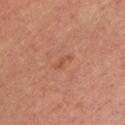No biopsy was performed on this lesion — it was imaged during a full skin examination and was not determined to be concerning. The lesion-visualizer software estimated a lesion area of about 3.5 mm², a shape eccentricity near 0.9, and two-axis asymmetry of about 0.4. The software also gave roughly 4 lightness units darker than nearby skin and a lesion-to-skin contrast of about 4.5 (normalized; higher = more distinct). The software also gave an automated nevus-likeness rating near 0 out of 100. The lesion is located on the front of the torso. The patient is a male about 30 years old. Cropped from a total-body skin-imaging series; the visible field is about 15 mm. The recorded lesion diameter is about 3 mm. This is a cross-polarized tile.